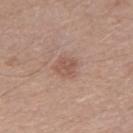Captured under white-light illumination. A roughly 15 mm field-of-view crop from a total-body skin photograph. Automated image analysis of the tile measured a mean CIELAB color near L≈54 a*≈20 b*≈27 and about 8 CIELAB-L* units darker than the surrounding skin. And it measured internal color variation of about 2 on a 0–10 scale and a peripheral color-asymmetry measure near 1. A male subject about 30 years old.